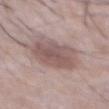| field | value |
|---|---|
| TBP lesion metrics | a mean CIELAB color near L≈54 a*≈17 b*≈19 and roughly 10 lightness units darker than nearby skin; a border-irregularity rating of about 2/10, a within-lesion color-variation index near 3/10, and radial color variation of about 1 |
| patient | male, approximately 65 years of age |
| acquisition | total-body-photography crop, ~15 mm field of view |
| lighting | white-light |
| location | the abdomen |
| diameter | ≈5.5 mm |